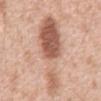Captured during whole-body skin photography for melanoma surveillance; the lesion was not biopsied.
A male subject, about 60 years old.
Automated tile analysis of the lesion measured an average lesion color of about L≈60 a*≈21 b*≈29 (CIELAB) and a lesion-to-skin contrast of about 7.5 (normalized; higher = more distinct). The software also gave a border-irregularity rating of about 6/10, a color-variation rating of about 8/10, and radial color variation of about 3. The analysis additionally found a classifier nevus-likeness of about 55/100 and a lesion-detection confidence of about 100/100.
The recorded lesion diameter is about 12 mm.
A 15 mm crop from a total-body photograph taken for skin-cancer surveillance.
From the abdomen.